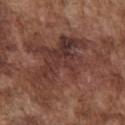biopsy_status: not biopsied; imaged during a skin examination
image:
  source: total-body photography crop
  field_of_view_mm: 15
patient:
  sex: male
  age_approx: 75
lesion_size:
  long_diameter_mm_approx: 12.0
lighting: white-light
site: chest